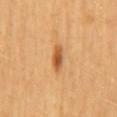Q: Is there a histopathology result?
A: no biopsy performed (imaged during a skin exam)
Q: How was the tile lit?
A: cross-polarized illumination
Q: What are the patient's age and sex?
A: female, approximately 60 years of age
Q: Lesion size?
A: about 3.5 mm
Q: What is the anatomic site?
A: the mid back
Q: What is the imaging modality?
A: ~15 mm tile from a whole-body skin photo